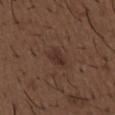No biopsy was performed on this lesion — it was imaged during a full skin examination and was not determined to be concerning.
About 3 mm across.
A close-up tile cropped from a whole-body skin photograph, about 15 mm across.
This is a white-light tile.
The patient is a male approximately 50 years of age.
The lesion is on the chest.